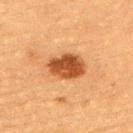Imaged during a routine full-body skin examination; the lesion was not biopsied and no histopathology is available.